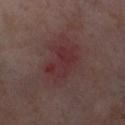| field | value |
|---|---|
| follow-up | total-body-photography surveillance lesion; no biopsy |
| patient | female, aged 53 to 57 |
| lesion size | ~4 mm (longest diameter) |
| illumination | cross-polarized illumination |
| image-analysis metrics | an average lesion color of about L≈31 a*≈23 b*≈17 (CIELAB), a lesion–skin lightness drop of about 6, and a normalized lesion–skin contrast near 5.5 |
| image source | 15 mm crop, total-body photography |
| location | the right thigh |